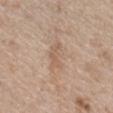follow-up — imaged on a skin check; not biopsied